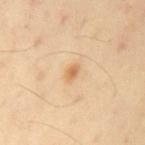Part of a total-body skin-imaging series; this lesion was reviewed on a skin check and was not flagged for biopsy. Captured under cross-polarized illumination. The lesion is located on the mid back. Automated tile analysis of the lesion measured a footprint of about 2 mm², an outline eccentricity of about 0.8 (0 = round, 1 = elongated), and a shape-asymmetry score of about 0.3 (0 = symmetric). The software also gave an average lesion color of about L≈67 a*≈20 b*≈41 (CIELAB), a lesion–skin lightness drop of about 10, and a normalized border contrast of about 7. The software also gave an automated nevus-likeness rating near 65 out of 100 and lesion-presence confidence of about 100/100. A male patient aged approximately 55. A roughly 15 mm field-of-view crop from a total-body skin photograph. The lesion's longest dimension is about 2 mm.